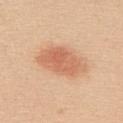Impression: Part of a total-body skin-imaging series; this lesion was reviewed on a skin check and was not flagged for biopsy. Clinical summary: A 15 mm close-up extracted from a 3D total-body photography capture. This is a white-light tile. A female subject aged approximately 20. The lesion is located on the back. Measured at roughly 5.5 mm in maximum diameter.